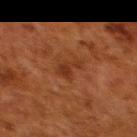Notes:
- biopsy status · imaged on a skin check; not biopsied
- subject · female, roughly 50 years of age
- illumination · cross-polarized
- acquisition · 15 mm crop, total-body photography
- site · the back
- diameter · ~3.5 mm (longest diameter)